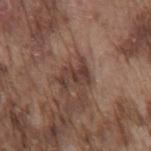This lesion was catalogued during total-body skin photography and was not selected for biopsy.
A 15 mm close-up extracted from a 3D total-body photography capture.
Automated tile analysis of the lesion measured a footprint of about 6 mm², a shape eccentricity near 0.75, and two-axis asymmetry of about 0.45. The software also gave a border-irregularity rating of about 6.5/10, a color-variation rating of about 2.5/10, and peripheral color asymmetry of about 1.
The lesion is located on the mid back.
A male subject roughly 75 years of age.
Approximately 3.5 mm at its widest.